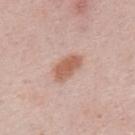biopsy_status: not biopsied; imaged during a skin examination
lighting: white-light
patient:
  sex: male
  age_approx: 25
site: upper back
lesion_size:
  long_diameter_mm_approx: 4.0
image:
  source: total-body photography crop
  field_of_view_mm: 15